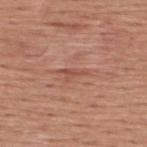The lesion's longest dimension is about 3.5 mm.
A male subject, approximately 45 years of age.
This is a white-light tile.
A region of skin cropped from a whole-body photographic capture, roughly 15 mm wide.
From the upper back.
The total-body-photography lesion software estimated a footprint of about 3 mm², a shape eccentricity near 0.9, and a symmetry-axis asymmetry near 0.55.